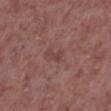* workup: imaged on a skin check; not biopsied
* subject: male, aged 53 to 57
* tile lighting: white-light
* site: the right lower leg
* automated metrics: an area of roughly 3 mm², a shape eccentricity near 0.85, and two-axis asymmetry of about 0.45; a border-irregularity index near 5/10, internal color variation of about 1.5 on a 0–10 scale, and a peripheral color-asymmetry measure near 0.5; an automated nevus-likeness rating near 0 out of 100 and a lesion-detection confidence of about 100/100
* acquisition: total-body-photography crop, ~15 mm field of view
* diameter: ≈2.5 mm